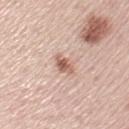Notes:
- biopsy status · imaged on a skin check; not biopsied
- patient · female, aged 58 to 62
- anatomic site · the left upper arm
- imaging modality · total-body-photography crop, ~15 mm field of view
- size · about 2.5 mm
- lighting · white-light